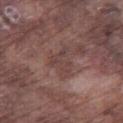Q: Is there a histopathology result?
A: total-body-photography surveillance lesion; no biopsy
Q: Lesion location?
A: the right thigh
Q: Who is the patient?
A: male, approximately 75 years of age
Q: How was this image acquired?
A: ~15 mm crop, total-body skin-cancer survey
Q: Illumination type?
A: white-light
Q: How large is the lesion?
A: ≈3.5 mm